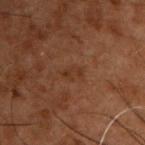Impression: Imaged during a routine full-body skin examination; the lesion was not biopsied and no histopathology is available. Acquisition and patient details: Imaged with cross-polarized lighting. From the upper back. A male patient in their 50s. Longest diameter approximately 2.5 mm. This image is a 15 mm lesion crop taken from a total-body photograph. Automated tile analysis of the lesion measured a lesion color around L≈25 a*≈17 b*≈24 in CIELAB, a lesion–skin lightness drop of about 4, and a lesion-to-skin contrast of about 5 (normalized; higher = more distinct). The software also gave border irregularity of about 4.5 on a 0–10 scale, a within-lesion color-variation index near 0/10, and a peripheral color-asymmetry measure near 0. And it measured an automated nevus-likeness rating near 0 out of 100 and lesion-presence confidence of about 100/100.